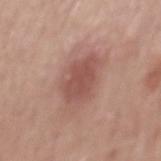notes: catalogued during a skin exam; not biopsied
patient: male, about 50 years old
diameter: about 5 mm
location: the mid back
image: 15 mm crop, total-body photography
image-analysis metrics: a lesion area of about 12 mm², a shape eccentricity near 0.8, and a shape-asymmetry score of about 0.2 (0 = symmetric); a border-irregularity rating of about 2.5/10, a within-lesion color-variation index near 2/10, and a peripheral color-asymmetry measure near 0.5; a classifier nevus-likeness of about 65/100 and a detector confidence of about 100 out of 100 that the crop contains a lesion